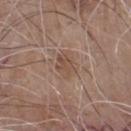Q: Was this lesion biopsied?
A: no biopsy performed (imaged during a skin exam)
Q: Lesion location?
A: the chest
Q: Patient demographics?
A: male, in their 80s
Q: What kind of image is this?
A: 15 mm crop, total-body photography